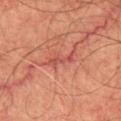| key | value |
|---|---|
| workup | total-body-photography surveillance lesion; no biopsy |
| patient | male, approximately 65 years of age |
| body site | the chest |
| image source | 15 mm crop, total-body photography |
| tile lighting | cross-polarized |